A 15 mm close-up extracted from a 3D total-body photography capture; the tile uses white-light illumination; the lesion is on the mid back; a female patient aged around 75; measured at roughly 10.5 mm in maximum diameter:
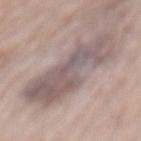Diagnosis: The lesion was biopsied, and histopathology showed an invasive melanoma; Breslow depth 0.4 mm, mitotic rate 1/mm².